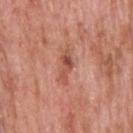Imaged during a routine full-body skin examination; the lesion was not biopsied and no histopathology is available.
The total-body-photography lesion software estimated an area of roughly 4 mm², an eccentricity of roughly 0.95, and two-axis asymmetry of about 0.4. And it measured a nevus-likeness score of about 0/100.
Cropped from a total-body skin-imaging series; the visible field is about 15 mm.
From the head or neck.
A male patient, approximately 60 years of age.
Imaged with white-light lighting.
Measured at roughly 4 mm in maximum diameter.